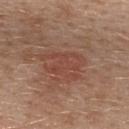Part of a total-body skin-imaging series; this lesion was reviewed on a skin check and was not flagged for biopsy.
The subject is a female aged 38 to 42.
The lesion is on the upper back.
Cropped from a whole-body photographic skin survey; the tile spans about 15 mm.
The recorded lesion diameter is about 5.5 mm.
The lesion-visualizer software estimated a footprint of about 11 mm² and a shape eccentricity near 0.75. It also reported a border-irregularity index near 6.5/10, a color-variation rating of about 2/10, and radial color variation of about 0.5. And it measured a classifier nevus-likeness of about 40/100 and lesion-presence confidence of about 100/100.
The tile uses white-light illumination.